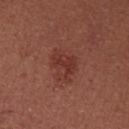{
  "biopsy_status": "not biopsied; imaged during a skin examination",
  "site": "right upper arm",
  "image": {
    "source": "total-body photography crop",
    "field_of_view_mm": 15
  },
  "patient": {
    "sex": "female",
    "age_approx": 25
  },
  "lighting": "white-light"
}